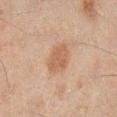Assessment:
Captured during whole-body skin photography for melanoma surveillance; the lesion was not biopsied.
Acquisition and patient details:
An algorithmic analysis of the crop reported an area of roughly 8 mm², an outline eccentricity of about 0.7 (0 = round, 1 = elongated), and two-axis asymmetry of about 0.15. It also reported a mean CIELAB color near L≈47 a*≈16 b*≈28 and a lesion-to-skin contrast of about 6.5 (normalized; higher = more distinct). The analysis additionally found border irregularity of about 1.5 on a 0–10 scale, internal color variation of about 1.5 on a 0–10 scale, and radial color variation of about 0.5. The software also gave a detector confidence of about 100 out of 100 that the crop contains a lesion. A male subject roughly 45 years of age. The recorded lesion diameter is about 4 mm. Captured under cross-polarized illumination. Located on the right lower leg. A 15 mm crop from a total-body photograph taken for skin-cancer surveillance.